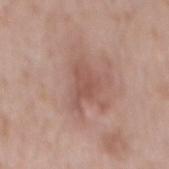This lesion was catalogued during total-body skin photography and was not selected for biopsy. A roughly 15 mm field-of-view crop from a total-body skin photograph. The recorded lesion diameter is about 5 mm. A male subject aged around 50. On the mid back. Captured under white-light illumination.